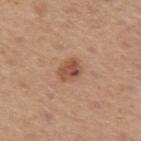Q: Is there a histopathology result?
A: catalogued during a skin exam; not biopsied
Q: What are the patient's age and sex?
A: male, aged around 75
Q: What lighting was used for the tile?
A: white-light
Q: What kind of image is this?
A: ~15 mm tile from a whole-body skin photo
Q: What did automated image analysis measure?
A: a lesion area of about 5 mm² and an outline eccentricity of about 0.65 (0 = round, 1 = elongated); a border-irregularity rating of about 2/10, a within-lesion color-variation index near 4.5/10, and peripheral color asymmetry of about 1.5
Q: Lesion location?
A: the left upper arm
Q: How large is the lesion?
A: ≈3 mm